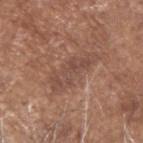Recorded during total-body skin imaging; not selected for excision or biopsy. A male subject in their 80s. The lesion is on the head or neck. Captured under white-light illumination. Cropped from a whole-body photographic skin survey; the tile spans about 15 mm. Measured at roughly 6.5 mm in maximum diameter.